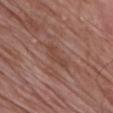  patient:
    sex: male
    age_approx: 65
  site: chest
  image:
    source: total-body photography crop
    field_of_view_mm: 15